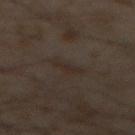Captured during whole-body skin photography for melanoma surveillance; the lesion was not biopsied. Automated image analysis of the tile measured an area of roughly 3.5 mm², a shape eccentricity near 0.95, and a shape-asymmetry score of about 0.4 (0 = symmetric). And it measured a lesion color around L≈23 a*≈9 b*≈16 in CIELAB, about 4 CIELAB-L* units darker than the surrounding skin, and a normalized lesion–skin contrast near 5.5. The software also gave a border-irregularity rating of about 5.5/10, a color-variation rating of about 0/10, and a peripheral color-asymmetry measure near 0. And it measured a detector confidence of about 90 out of 100 that the crop contains a lesion. A 15 mm close-up tile from a total-body photography series done for melanoma screening. A male patient aged around 60. The lesion's longest dimension is about 3.5 mm. This is a cross-polarized tile. Located on the abdomen.